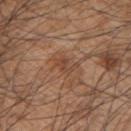Acquisition and patient details: Cropped from a whole-body photographic skin survey; the tile spans about 15 mm. The subject is a male in their mid- to late 40s. From the upper back. Captured under white-light illumination.